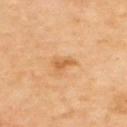The lesion was photographed on a routine skin check and not biopsied; there is no pathology result.